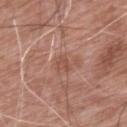Q: Is there a histopathology result?
A: no biopsy performed (imaged during a skin exam)
Q: How was the tile lit?
A: white-light
Q: What is the anatomic site?
A: the upper back
Q: What kind of image is this?
A: ~15 mm crop, total-body skin-cancer survey
Q: Who is the patient?
A: male, about 60 years old
Q: Lesion size?
A: about 2.5 mm